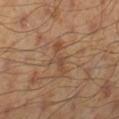Assessment: Imaged during a routine full-body skin examination; the lesion was not biopsied and no histopathology is available. Clinical summary: A male subject, aged 43–47. On the left lower leg. A roughly 15 mm field-of-view crop from a total-body skin photograph. The total-body-photography lesion software estimated a lesion area of about 6.5 mm², a shape eccentricity near 0.9, and a symmetry-axis asymmetry near 0.45. The analysis additionally found border irregularity of about 6.5 on a 0–10 scale, a color-variation rating of about 2.5/10, and radial color variation of about 0.5. The lesion's longest dimension is about 4.5 mm. This is a cross-polarized tile.